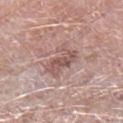subject: male, approximately 50 years of age
site: the left lower leg
image: ~15 mm crop, total-body skin-cancer survey
TBP lesion metrics: an average lesion color of about L≈54 a*≈20 b*≈22 (CIELAB), about 10 CIELAB-L* units darker than the surrounding skin, and a normalized border contrast of about 7; border irregularity of about 7 on a 0–10 scale and peripheral color asymmetry of about 1; a classifier nevus-likeness of about 0/100 and a lesion-detection confidence of about 100/100